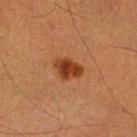Imaged during a routine full-body skin examination; the lesion was not biopsied and no histopathology is available. A male patient aged 38–42. Approximately 3.5 mm at its widest. A close-up tile cropped from a whole-body skin photograph, about 15 mm across. The lesion-visualizer software estimated an area of roughly 6 mm², a shape eccentricity near 0.75, and a symmetry-axis asymmetry near 0.25. The analysis additionally found a lesion-detection confidence of about 100/100. The lesion is located on the right lower leg. This is a cross-polarized tile.